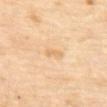Q: Was this lesion biopsied?
A: imaged on a skin check; not biopsied
Q: Lesion size?
A: about 2.5 mm
Q: What is the anatomic site?
A: the upper back
Q: Illumination type?
A: cross-polarized illumination
Q: What are the patient's age and sex?
A: female, aged 58 to 62
Q: What is the imaging modality?
A: ~15 mm crop, total-body skin-cancer survey